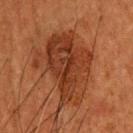Notes:
• follow-up · catalogued during a skin exam; not biopsied
• lesion diameter · ≈8 mm
• automated lesion analysis · a lesion color around L≈29 a*≈22 b*≈28 in CIELAB, roughly 8 lightness units darker than nearby skin, and a lesion-to-skin contrast of about 8 (normalized; higher = more distinct); a border-irregularity rating of about 5/10, a within-lesion color-variation index near 5.5/10, and radial color variation of about 2
• imaging modality · 15 mm crop, total-body photography
• subject · male, in their 50s
• lighting · cross-polarized illumination
• anatomic site · the upper back